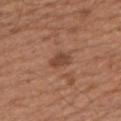Part of a total-body skin-imaging series; this lesion was reviewed on a skin check and was not flagged for biopsy. The patient is a male approximately 65 years of age. This is a white-light tile. A close-up tile cropped from a whole-body skin photograph, about 15 mm across. From the arm. Approximately 3 mm at its widest.